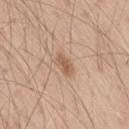Captured during whole-body skin photography for melanoma surveillance; the lesion was not biopsied. The total-body-photography lesion software estimated roughly 10 lightness units darker than nearby skin and a lesion-to-skin contrast of about 7 (normalized; higher = more distinct). It also reported a border-irregularity index near 2/10 and a peripheral color-asymmetry measure near 1. And it measured a nevus-likeness score of about 90/100 and lesion-presence confidence of about 100/100. A male patient, aged approximately 60. Imaged with white-light lighting. A 15 mm crop from a total-body photograph taken for skin-cancer surveillance. Located on the right thigh.